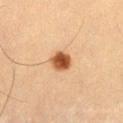follow-up: total-body-photography surveillance lesion; no biopsy | TBP lesion metrics: a mean CIELAB color near L≈44 a*≈22 b*≈34, a lesion–skin lightness drop of about 17, and a normalized border contrast of about 12.5; a border-irregularity index near 1.5/10, a color-variation rating of about 4.5/10, and peripheral color asymmetry of about 1.5 | site: the left thigh | subject: male, aged 58–62 | image: ~15 mm crop, total-body skin-cancer survey.